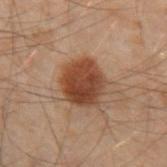Clinical impression: Part of a total-body skin-imaging series; this lesion was reviewed on a skin check and was not flagged for biopsy. Context: About 4.5 mm across. This image is a 15 mm lesion crop taken from a total-body photograph. Imaged with cross-polarized lighting. A male subject, aged approximately 50. The lesion is located on the left forearm.